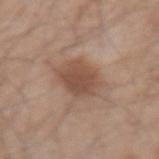<case>
<biopsy_status>not biopsied; imaged during a skin examination</biopsy_status>
<patient>
  <sex>male</sex>
  <age_approx>45</age_approx>
</patient>
<image>
  <source>total-body photography crop</source>
  <field_of_view_mm>15</field_of_view_mm>
</image>
<automated_metrics>
  <area_mm2_approx>11.0</area_mm2_approx>
  <eccentricity>0.55</eccentricity>
  <shape_asymmetry>0.15</shape_asymmetry>
  <nevus_likeness_0_100>90</nevus_likeness_0_100>
</automated_metrics>
<site>arm</site>
</case>